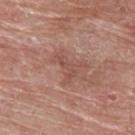Impression:
No biopsy was performed on this lesion — it was imaged during a full skin examination and was not determined to be concerning.
Context:
A roughly 15 mm field-of-view crop from a total-body skin photograph. From the upper back. A male patient aged around 65. The total-body-photography lesion software estimated an outline eccentricity of about 0.9 (0 = round, 1 = elongated). The software also gave a lesion color around L≈50 a*≈23 b*≈26 in CIELAB and about 7 CIELAB-L* units darker than the surrounding skin. The analysis additionally found a detector confidence of about 100 out of 100 that the crop contains a lesion. Approximately 3 mm at its widest. The tile uses white-light illumination.